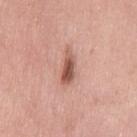Q: What is the anatomic site?
A: the left thigh
Q: What is the lesion's diameter?
A: about 4.5 mm
Q: How was this image acquired?
A: ~15 mm crop, total-body skin-cancer survey
Q: What lighting was used for the tile?
A: white-light illumination
Q: Patient demographics?
A: female, roughly 50 years of age